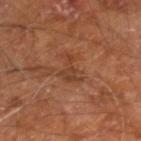workup: total-body-photography surveillance lesion; no biopsy
lesion diameter: ~3.5 mm (longest diameter)
TBP lesion metrics: a lesion area of about 5 mm², a shape eccentricity near 0.8, and a symmetry-axis asymmetry near 0.6; about 7 CIELAB-L* units darker than the surrounding skin and a normalized lesion–skin contrast near 5.5; lesion-presence confidence of about 100/100
body site: the right leg
patient: male, in their 60s
imaging modality: ~15 mm crop, total-body skin-cancer survey